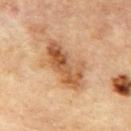Case summary:
– notes: catalogued during a skin exam; not biopsied
– subject: male, aged 83 or over
– TBP lesion metrics: an eccentricity of roughly 0.9 and a shape-asymmetry score of about 0.3 (0 = symmetric); an average lesion color of about L≈55 a*≈22 b*≈37 (CIELAB), about 13 CIELAB-L* units darker than the surrounding skin, and a normalized lesion–skin contrast near 8.5; a classifier nevus-likeness of about 35/100 and a lesion-detection confidence of about 100/100
– acquisition: 15 mm crop, total-body photography
– lighting: cross-polarized
– location: the upper back